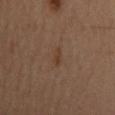{
  "site": "mid back",
  "lesion_size": {
    "long_diameter_mm_approx": 2.5
  },
  "patient": {
    "sex": "male",
    "age_approx": 60
  },
  "image": {
    "source": "total-body photography crop",
    "field_of_view_mm": 15
  },
  "lighting": "cross-polarized"
}